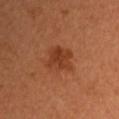{
  "biopsy_status": "not biopsied; imaged during a skin examination"
}